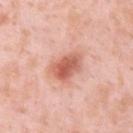Q: Was this lesion biopsied?
A: imaged on a skin check; not biopsied
Q: What is the anatomic site?
A: the upper back
Q: How was this image acquired?
A: total-body-photography crop, ~15 mm field of view
Q: How large is the lesion?
A: ~4.5 mm (longest diameter)
Q: How was the tile lit?
A: white-light
Q: Who is the patient?
A: male, approximately 35 years of age
Q: Automated lesion metrics?
A: a footprint of about 9.5 mm², an eccentricity of roughly 0.75, and two-axis asymmetry of about 0.25; an automated nevus-likeness rating near 95 out of 100 and a lesion-detection confidence of about 100/100